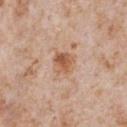Assessment: Captured during whole-body skin photography for melanoma surveillance; the lesion was not biopsied. Acquisition and patient details: The subject is a male roughly 65 years of age. Longest diameter approximately 3 mm. Imaged with white-light lighting. Automated image analysis of the tile measured a lesion color around L≈58 a*≈21 b*≈34 in CIELAB, about 10 CIELAB-L* units darker than the surrounding skin, and a lesion-to-skin contrast of about 8 (normalized; higher = more distinct). And it measured a classifier nevus-likeness of about 90/100 and lesion-presence confidence of about 100/100. This image is a 15 mm lesion crop taken from a total-body photograph. Located on the chest.